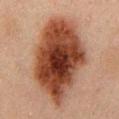Q: Is there a histopathology result?
A: no biopsy performed (imaged during a skin exam)
Q: What is the lesion's diameter?
A: ~11.5 mm (longest diameter)
Q: How was the tile lit?
A: cross-polarized
Q: Who is the patient?
A: male, in their 50s
Q: What kind of image is this?
A: ~15 mm crop, total-body skin-cancer survey
Q: Where on the body is the lesion?
A: the chest